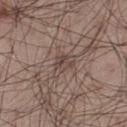lesion diameter: about 2.5 mm
subject: male, about 55 years old
TBP lesion metrics: a shape eccentricity near 0.6 and a shape-asymmetry score of about 0.35 (0 = symmetric); a border-irregularity rating of about 3.5/10, a within-lesion color-variation index near 4.5/10, and radial color variation of about 2; a nevus-likeness score of about 0/100 and lesion-presence confidence of about 85/100
tile lighting: white-light
site: the left thigh
acquisition: ~15 mm crop, total-body skin-cancer survey